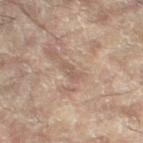workup — total-body-photography surveillance lesion; no biopsy | anatomic site — the left leg | lesion size — ~2.5 mm (longest diameter) | subject — female, roughly 80 years of age | image — ~15 mm crop, total-body skin-cancer survey | illumination — cross-polarized.